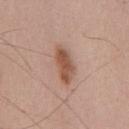Q: Was a biopsy performed?
A: total-body-photography surveillance lesion; no biopsy
Q: Automated lesion metrics?
A: a lesion area of about 8 mm² and a symmetry-axis asymmetry near 0.25; a classifier nevus-likeness of about 95/100 and a lesion-detection confidence of about 100/100
Q: Illumination type?
A: white-light
Q: Patient demographics?
A: male, aged 63–67
Q: What is the imaging modality?
A: ~15 mm crop, total-body skin-cancer survey
Q: What is the anatomic site?
A: the chest
Q: How large is the lesion?
A: ~5 mm (longest diameter)